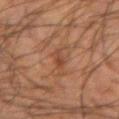biopsy status=no biopsy performed (imaged during a skin exam)
lighting=cross-polarized illumination
image source=~15 mm crop, total-body skin-cancer survey
diameter=about 3 mm
subject=male, in their mid-60s
location=the left forearm